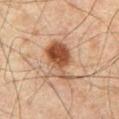The lesion was photographed on a routine skin check and not biopsied; there is no pathology result.
An algorithmic analysis of the crop reported a border-irregularity index near 4/10, internal color variation of about 9 on a 0–10 scale, and a peripheral color-asymmetry measure near 3.5.
The lesion is on the abdomen.
This is a cross-polarized tile.
A close-up tile cropped from a whole-body skin photograph, about 15 mm across.
A male subject aged approximately 65.
About 5.5 mm across.